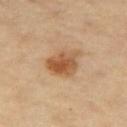The lesion is located on the right thigh.
A 15 mm close-up tile from a total-body photography series done for melanoma screening.
The tile uses cross-polarized illumination.
Longest diameter approximately 4.5 mm.
A female patient about 70 years old.